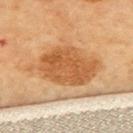follow-up — total-body-photography surveillance lesion; no biopsy | subject — about 70 years old | imaging modality — total-body-photography crop, ~15 mm field of view | lighting — cross-polarized illumination | lesion diameter — ~8 mm (longest diameter) | anatomic site — the upper back.